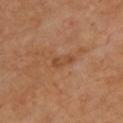Impression:
This lesion was catalogued during total-body skin photography and was not selected for biopsy.
Acquisition and patient details:
On the upper back. A female subject, approximately 70 years of age. About 3 mm across. This is a cross-polarized tile. A region of skin cropped from a whole-body photographic capture, roughly 15 mm wide. The total-body-photography lesion software estimated a footprint of about 3.5 mm², a shape eccentricity near 0.85, and a symmetry-axis asymmetry near 0.4. It also reported a lesion color around L≈45 a*≈21 b*≈34 in CIELAB, a lesion–skin lightness drop of about 6, and a normalized lesion–skin contrast near 5.5. And it measured a classifier nevus-likeness of about 0/100.